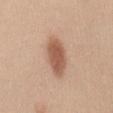follow-up=no biopsy performed (imaged during a skin exam)
lesion size=about 5.5 mm
subject=female, aged 43 to 47
body site=the mid back
image-analysis metrics=an area of roughly 11 mm² and a symmetry-axis asymmetry near 0.15; a classifier nevus-likeness of about 100/100 and a detector confidence of about 100 out of 100 that the crop contains a lesion
acquisition=15 mm crop, total-body photography